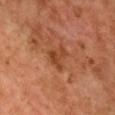Clinical impression: This lesion was catalogued during total-body skin photography and was not selected for biopsy. Image and clinical context: The total-body-photography lesion software estimated a normalized lesion–skin contrast near 6.5. The software also gave a nevus-likeness score of about 0/100. The lesion is on the front of the torso. A region of skin cropped from a whole-body photographic capture, roughly 15 mm wide. The patient is a female aged approximately 60. Captured under cross-polarized illumination.